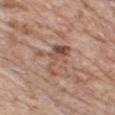| key | value |
|---|---|
| biopsy status | imaged on a skin check; not biopsied |
| body site | the chest |
| acquisition | ~15 mm tile from a whole-body skin photo |
| subject | male, about 75 years old |
| diameter | about 5 mm |